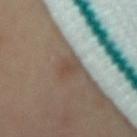  lesion_size:
    long_diameter_mm_approx: 3.0
  image:
    source: total-body photography crop
    field_of_view_mm: 15
  automated_metrics:
    nevus_likeness_0_100: 90
  lighting: cross-polarized
  site: mid back